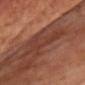follow-up = catalogued during a skin exam; not biopsied
image source = ~15 mm crop, total-body skin-cancer survey
patient = male, about 65 years old
anatomic site = the chest
lesion diameter = ≈5 mm